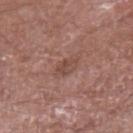The lesion was tiled from a total-body skin photograph and was not biopsied. Approximately 3.5 mm at its widest. The tile uses white-light illumination. The lesion is on the left upper arm. A male subject aged around 55. A region of skin cropped from a whole-body photographic capture, roughly 15 mm wide.